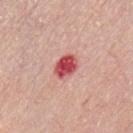Recorded during total-body skin imaging; not selected for excision or biopsy. A 15 mm close-up tile from a total-body photography series done for melanoma screening. The patient is a male aged 58 to 62. On the chest.